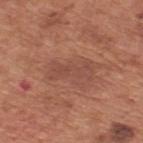The lesion was tiled from a total-body skin photograph and was not biopsied.
A male subject, aged 63 to 67.
The lesion's longest dimension is about 5 mm.
The lesion is on the upper back.
Captured under white-light illumination.
A close-up tile cropped from a whole-body skin photograph, about 15 mm across.